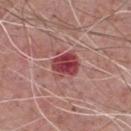Captured during whole-body skin photography for melanoma surveillance; the lesion was not biopsied.
Cropped from a total-body skin-imaging series; the visible field is about 15 mm.
A male subject aged 73 to 77.
The lesion is on the chest.
This is a white-light tile.
Approximately 3.5 mm at its widest.